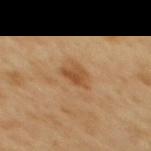This lesion was catalogued during total-body skin photography and was not selected for biopsy. Automated tile analysis of the lesion measured an average lesion color of about L≈50 a*≈21 b*≈38 (CIELAB) and roughly 9 lightness units darker than nearby skin. The analysis additionally found a classifier nevus-likeness of about 35/100 and a lesion-detection confidence of about 100/100. On the mid back. A male subject in their 50s. Cropped from a whole-body photographic skin survey; the tile spans about 15 mm. Captured under cross-polarized illumination. About 2.5 mm across.